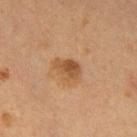No biopsy was performed on this lesion — it was imaged during a full skin examination and was not determined to be concerning.
An algorithmic analysis of the crop reported a classifier nevus-likeness of about 10/100 and a detector confidence of about 100 out of 100 that the crop contains a lesion.
A 15 mm crop from a total-body photograph taken for skin-cancer surveillance.
Captured under cross-polarized illumination.
On the abdomen.
About 3 mm across.
A male patient aged around 55.